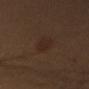| key | value |
|---|---|
| workup | imaged on a skin check; not biopsied |
| location | the arm |
| illumination | cross-polarized illumination |
| lesion diameter | ≈3.5 mm |
| image-analysis metrics | a symmetry-axis asymmetry near 0.25; an average lesion color of about L≈24 a*≈15 b*≈22 (CIELAB), about 4 CIELAB-L* units darker than the surrounding skin, and a lesion-to-skin contrast of about 5.5 (normalized; higher = more distinct); a border-irregularity index near 2.5/10, a color-variation rating of about 2/10, and peripheral color asymmetry of about 0.5; an automated nevus-likeness rating near 50 out of 100 |
| patient | female, aged approximately 30 |
| acquisition | ~15 mm crop, total-body skin-cancer survey |